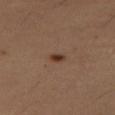Clinical summary:
A female subject, aged 53–57. The lesion is located on the leg. The tile uses cross-polarized illumination. This image is a 15 mm lesion crop taken from a total-body photograph.